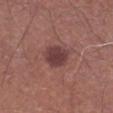notes=catalogued during a skin exam; not biopsied
anatomic site=the leg
subject=male, aged 68 to 72
imaging modality=~15 mm crop, total-body skin-cancer survey